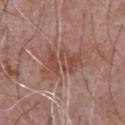follow-up — no biopsy performed (imaged during a skin exam) | image source — ~15 mm tile from a whole-body skin photo | patient — male, aged around 70 | TBP lesion metrics — a shape eccentricity near 0.8 and a shape-asymmetry score of about 0.4 (0 = symmetric); an average lesion color of about L≈49 a*≈21 b*≈26 (CIELAB), a lesion–skin lightness drop of about 7, and a normalized lesion–skin contrast near 6; border irregularity of about 7 on a 0–10 scale and peripheral color asymmetry of about 1 | site — the chest.